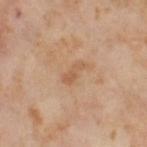Q: Was a biopsy performed?
A: total-body-photography surveillance lesion; no biopsy
Q: What is the imaging modality?
A: ~15 mm tile from a whole-body skin photo
Q: What lighting was used for the tile?
A: cross-polarized
Q: Where on the body is the lesion?
A: the right thigh
Q: Who is the patient?
A: female, roughly 55 years of age
Q: Lesion size?
A: ~3 mm (longest diameter)